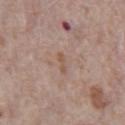Q: Was a biopsy performed?
A: catalogued during a skin exam; not biopsied
Q: Lesion location?
A: the abdomen
Q: What are the patient's age and sex?
A: male, aged 68 to 72
Q: What is the imaging modality?
A: ~15 mm tile from a whole-body skin photo